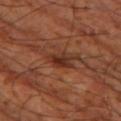Clinical impression:
Recorded during total-body skin imaging; not selected for excision or biopsy.
Acquisition and patient details:
Measured at roughly 3 mm in maximum diameter. The lesion-visualizer software estimated a border-irregularity rating of about 3/10 and internal color variation of about 5.5 on a 0–10 scale. The analysis additionally found an automated nevus-likeness rating near 30 out of 100 and a detector confidence of about 100 out of 100 that the crop contains a lesion. Located on the left thigh. This is a cross-polarized tile. A patient in their mid- to late 60s. A lesion tile, about 15 mm wide, cut from a 3D total-body photograph.